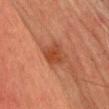Case summary:
- follow-up · catalogued during a skin exam; not biopsied
- automated lesion analysis · a footprint of about 7.5 mm², an eccentricity of roughly 0.65, and a shape-asymmetry score of about 0.2 (0 = symmetric); a mean CIELAB color near L≈38 a*≈24 b*≈31, about 8 CIELAB-L* units darker than the surrounding skin, and a normalized lesion–skin contrast near 7; a border-irregularity rating of about 2/10 and a color-variation rating of about 3.5/10
- lesion diameter · ~3.5 mm (longest diameter)
- imaging modality · total-body-photography crop, ~15 mm field of view
- illumination · cross-polarized
- site · the chest
- patient · male, in their mid- to late 40s